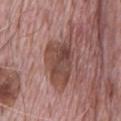Acquisition and patient details: A roughly 15 mm field-of-view crop from a total-body skin photograph. Captured under white-light illumination. The lesion-visualizer software estimated a lesion–skin lightness drop of about 11. It also reported a nevus-likeness score of about 0/100 and a detector confidence of about 85 out of 100 that the crop contains a lesion. A male subject, aged approximately 70. Located on the chest. The recorded lesion diameter is about 8 mm.